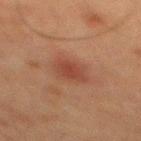Part of a total-body skin-imaging series; this lesion was reviewed on a skin check and was not flagged for biopsy. Captured under cross-polarized illumination. Approximately 2.5 mm at its widest. The subject is a male aged around 60. A close-up tile cropped from a whole-body skin photograph, about 15 mm across. Automated image analysis of the tile measured border irregularity of about 2 on a 0–10 scale, internal color variation of about 1.5 on a 0–10 scale, and peripheral color asymmetry of about 0.5. The analysis additionally found an automated nevus-likeness rating near 30 out of 100 and lesion-presence confidence of about 100/100. The lesion is located on the upper back.